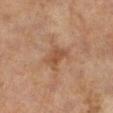Q: Was a biopsy performed?
A: imaged on a skin check; not biopsied
Q: How was this image acquired?
A: ~15 mm crop, total-body skin-cancer survey
Q: Who is the patient?
A: female, aged around 60
Q: Lesion size?
A: about 3 mm
Q: Where on the body is the lesion?
A: the right lower leg
Q: What did automated image analysis measure?
A: a lesion area of about 5.5 mm², an eccentricity of roughly 0.7, and a shape-asymmetry score of about 0.2 (0 = symmetric); a border-irregularity index near 2.5/10, a within-lesion color-variation index near 2/10, and peripheral color asymmetry of about 0.5